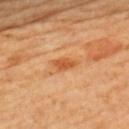{
  "lesion_size": {
    "long_diameter_mm_approx": 3.0
  },
  "image": {
    "source": "total-body photography crop",
    "field_of_view_mm": 15
  },
  "patient": {
    "sex": "female",
    "age_approx": 65
  },
  "site": "upper back",
  "lighting": "cross-polarized"
}